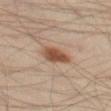This lesion was catalogued during total-body skin photography and was not selected for biopsy.
The lesion is on the left thigh.
The subject is a male roughly 40 years of age.
The lesion's longest dimension is about 4 mm.
Cropped from a total-body skin-imaging series; the visible field is about 15 mm.
The lesion-visualizer software estimated an area of roughly 7 mm² and two-axis asymmetry of about 0.2. It also reported a border-irregularity rating of about 2/10, a color-variation rating of about 3/10, and peripheral color asymmetry of about 1.